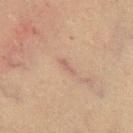Part of a total-body skin-imaging series; this lesion was reviewed on a skin check and was not flagged for biopsy. A close-up tile cropped from a whole-body skin photograph, about 15 mm across. On the chest. The patient is a male aged around 60.